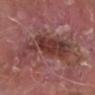Q: Was a biopsy performed?
A: imaged on a skin check; not biopsied
Q: What are the patient's age and sex?
A: male, about 65 years old
Q: What is the imaging modality?
A: ~15 mm tile from a whole-body skin photo
Q: How was the tile lit?
A: white-light illumination
Q: Lesion location?
A: the right lower leg
Q: Lesion size?
A: about 9 mm